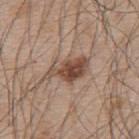biopsy_status: not biopsied; imaged during a skin examination
site: upper back
lesion_size:
  long_diameter_mm_approx: 5.5
lighting: white-light
automated_metrics:
  vs_skin_darker_L: 13.0
  vs_skin_contrast_norm: 9.5
  nevus_likeness_0_100: 90
  lesion_detection_confidence_0_100: 100
patient:
  sex: male
  age_approx: 55
image:
  source: total-body photography crop
  field_of_view_mm: 15